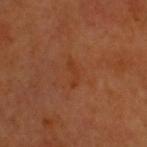image = total-body-photography crop, ~15 mm field of view | illumination = cross-polarized illumination | automated metrics = border irregularity of about 4 on a 0–10 scale, internal color variation of about 0 on a 0–10 scale, and a peripheral color-asymmetry measure near 0; a nevus-likeness score of about 0/100 and a detector confidence of about 100 out of 100 that the crop contains a lesion | size = ~3.5 mm (longest diameter) | location = the head or neck | subject = male, aged around 60.